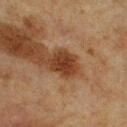Clinical impression: The lesion was tiled from a total-body skin photograph and was not biopsied. Acquisition and patient details: A male subject, roughly 75 years of age. Longest diameter approximately 3.5 mm. The tile uses cross-polarized illumination. A 15 mm close-up tile from a total-body photography series done for melanoma screening. The lesion is located on the chest.